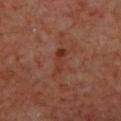| key | value |
|---|---|
| workup | imaged on a skin check; not biopsied |
| location | the upper back |
| lighting | cross-polarized |
| imaging modality | 15 mm crop, total-body photography |
| diameter | ~3.5 mm (longest diameter) |
| patient | male, aged approximately 70 |